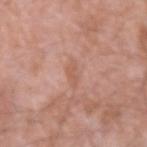Impression: The lesion was photographed on a routine skin check and not biopsied; there is no pathology result. Context: A region of skin cropped from a whole-body photographic capture, roughly 15 mm wide. The tile uses white-light illumination. Measured at roughly 3 mm in maximum diameter. The lesion is on the right forearm. A male subject aged around 60.